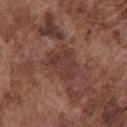* illumination · white-light
* site · the front of the torso
* patient · male, aged approximately 75
* imaging modality · 15 mm crop, total-body photography
* size · about 4.5 mm